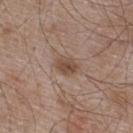Notes:
• follow-up: no biopsy performed (imaged during a skin exam)
• lighting: white-light illumination
• image: total-body-photography crop, ~15 mm field of view
• anatomic site: the upper back
• patient: male, in their mid- to late 50s
• image-analysis metrics: roughly 10 lightness units darker than nearby skin and a lesion-to-skin contrast of about 8 (normalized; higher = more distinct)